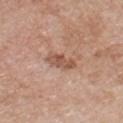Q: What did automated image analysis measure?
A: a shape eccentricity near 0.9 and a symmetry-axis asymmetry near 0.3; a mean CIELAB color near L≈54 a*≈21 b*≈29, about 10 CIELAB-L* units darker than the surrounding skin, and a normalized border contrast of about 7; a within-lesion color-variation index near 3/10 and a peripheral color-asymmetry measure near 1
Q: Where on the body is the lesion?
A: the chest
Q: How was this image acquired?
A: ~15 mm tile from a whole-body skin photo
Q: Lesion size?
A: ≈4 mm
Q: What lighting was used for the tile?
A: white-light
Q: Patient demographics?
A: male, approximately 70 years of age